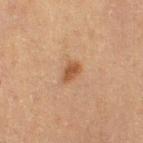Imaged during a routine full-body skin examination; the lesion was not biopsied and no histopathology is available.
Cropped from a whole-body photographic skin survey; the tile spans about 15 mm.
On the right thigh.
A female subject aged approximately 55.
Captured under cross-polarized illumination.